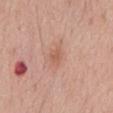No biopsy was performed on this lesion — it was imaged during a full skin examination and was not determined to be concerning. On the mid back. A region of skin cropped from a whole-body photographic capture, roughly 15 mm wide. A male patient, about 70 years old.